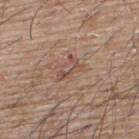follow-up = no biopsy performed (imaged during a skin exam)
subject = male, aged 63 to 67
illumination = white-light illumination
location = the back
acquisition = ~15 mm tile from a whole-body skin photo